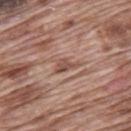Imaged during a routine full-body skin examination; the lesion was not biopsied and no histopathology is available. The lesion is on the mid back. A close-up tile cropped from a whole-body skin photograph, about 15 mm across. Measured at roughly 3 mm in maximum diameter. A male subject aged 68–72. This is a white-light tile.